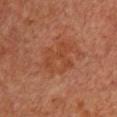follow-up: no biopsy performed (imaged during a skin exam) | image: ~15 mm crop, total-body skin-cancer survey | subject: female, approximately 50 years of age | body site: the chest.